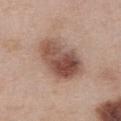Part of a total-body skin-imaging series; this lesion was reviewed on a skin check and was not flagged for biopsy.
This is a white-light tile.
An algorithmic analysis of the crop reported a lesion area of about 19 mm², an outline eccentricity of about 0.8 (0 = round, 1 = elongated), and two-axis asymmetry of about 0.2. And it measured border irregularity of about 2 on a 0–10 scale, a within-lesion color-variation index near 8/10, and a peripheral color-asymmetry measure near 3. And it measured lesion-presence confidence of about 100/100.
A female subject, aged around 40.
A 15 mm close-up extracted from a 3D total-body photography capture.
On the abdomen.